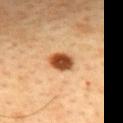biopsy status: total-body-photography surveillance lesion; no biopsy
acquisition: ~15 mm tile from a whole-body skin photo
anatomic site: the mid back
illumination: cross-polarized illumination
subject: male, aged 43–47